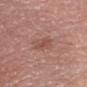Q: Was a biopsy performed?
A: no biopsy performed (imaged during a skin exam)
Q: What did automated image analysis measure?
A: a mean CIELAB color near L≈50 a*≈23 b*≈27 and a lesion-to-skin contrast of about 6 (normalized; higher = more distinct)
Q: Who is the patient?
A: male, aged 53–57
Q: What lighting was used for the tile?
A: white-light illumination
Q: How was this image acquired?
A: ~15 mm crop, total-body skin-cancer survey
Q: What is the anatomic site?
A: the head or neck